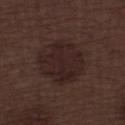{
  "biopsy_status": "not biopsied; imaged during a skin examination",
  "image": {
    "source": "total-body photography crop",
    "field_of_view_mm": 15
  },
  "patient": {
    "sex": "male",
    "age_approx": 70
  },
  "site": "left lower leg"
}